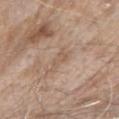{
  "biopsy_status": "not biopsied; imaged during a skin examination",
  "lesion_size": {
    "long_diameter_mm_approx": 3.0
  },
  "patient": {
    "sex": "female",
    "age_approx": "85+"
  },
  "image": {
    "source": "total-body photography crop",
    "field_of_view_mm": 15
  },
  "site": "right upper arm",
  "lighting": "white-light",
  "automated_metrics": {
    "border_irregularity_0_10": 7.5,
    "color_variation_0_10": 0.0,
    "peripheral_color_asymmetry": 0.0
  }
}